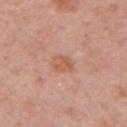{"biopsy_status": "not biopsied; imaged during a skin examination", "automated_metrics": {"area_mm2_approx": 4.5, "eccentricity": 0.6, "shape_asymmetry": 0.25, "cielab_L": 58, "cielab_a": 24, "cielab_b": 32, "vs_skin_darker_L": 8.0, "vs_skin_contrast_norm": 6.5, "border_irregularity_0_10": 2.0, "color_variation_0_10": 3.0, "peripheral_color_asymmetry": 1.0}, "lesion_size": {"long_diameter_mm_approx": 2.5}, "lighting": "white-light", "image": {"source": "total-body photography crop", "field_of_view_mm": 15}, "patient": {"sex": "female", "age_approx": 45}, "site": "right upper arm"}